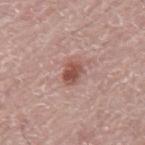follow-up=catalogued during a skin exam; not biopsied | diameter=≈3 mm | image source=15 mm crop, total-body photography | subject=male, aged approximately 75 | location=the back.